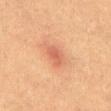Q: Is there a histopathology result?
A: catalogued during a skin exam; not biopsied
Q: Illumination type?
A: cross-polarized
Q: What is the lesion's diameter?
A: about 2.5 mm
Q: Patient demographics?
A: male, aged around 40
Q: What is the anatomic site?
A: the abdomen
Q: How was this image acquired?
A: 15 mm crop, total-body photography